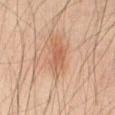{
  "biopsy_status": "not biopsied; imaged during a skin examination",
  "lesion_size": {
    "long_diameter_mm_approx": 4.5
  },
  "lighting": "cross-polarized",
  "patient": {
    "sex": "male",
    "age_approx": 40
  },
  "image": {
    "source": "total-body photography crop",
    "field_of_view_mm": 15
  },
  "site": "abdomen"
}